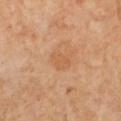Impression:
Imaged during a routine full-body skin examination; the lesion was not biopsied and no histopathology is available.
Context:
A lesion tile, about 15 mm wide, cut from a 3D total-body photograph. From the left lower leg. A female subject approximately 50 years of age. Imaged with cross-polarized lighting.